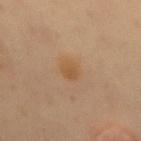The lesion was tiled from a total-body skin photograph and was not biopsied.